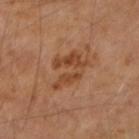Impression:
No biopsy was performed on this lesion — it was imaged during a full skin examination and was not determined to be concerning.
Clinical summary:
A female subject aged around 60. A 15 mm close-up tile from a total-body photography series done for melanoma screening. Automated tile analysis of the lesion measured a footprint of about 11 mm², an outline eccentricity of about 0.7 (0 = round, 1 = elongated), and two-axis asymmetry of about 0.5. The analysis additionally found an average lesion color of about L≈46 a*≈23 b*≈35 (CIELAB), about 8 CIELAB-L* units darker than the surrounding skin, and a lesion-to-skin contrast of about 7 (normalized; higher = more distinct). And it measured a border-irregularity rating of about 8.5/10, a within-lesion color-variation index near 3.5/10, and radial color variation of about 1. The software also gave a detector confidence of about 100 out of 100 that the crop contains a lesion. The lesion's longest dimension is about 5 mm. The lesion is located on the right forearm. Captured under cross-polarized illumination.